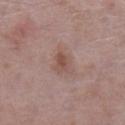The lesion was tiled from a total-body skin photograph and was not biopsied. Measured at roughly 2.5 mm in maximum diameter. A 15 mm close-up extracted from a 3D total-body photography capture. A female patient roughly 70 years of age. On the leg.